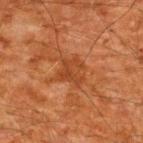biopsy status = catalogued during a skin exam; not biopsied.